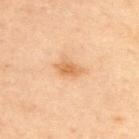Recorded during total-body skin imaging; not selected for excision or biopsy. A male subject in their mid-40s. Cropped from a total-body skin-imaging series; the visible field is about 15 mm. The tile uses cross-polarized illumination. The lesion is on the upper back. The lesion's longest dimension is about 3.5 mm.